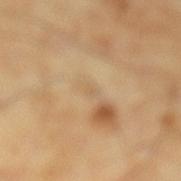  biopsy_status: not biopsied; imaged during a skin examination
  lesion_size:
    long_diameter_mm_approx: 1.5
  automated_metrics:
    area_mm2_approx: 1.0
    eccentricity: 0.75
  lighting: cross-polarized
  image:
    source: total-body photography crop
    field_of_view_mm: 15
  patient:
    sex: male
    age_approx: 55
  site: left lower leg